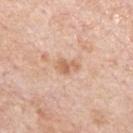No biopsy was performed on this lesion — it was imaged during a full skin examination and was not determined to be concerning. The subject is a male in their 70s. Imaged with white-light lighting. A region of skin cropped from a whole-body photographic capture, roughly 15 mm wide. Automated image analysis of the tile measured a within-lesion color-variation index near 2.5/10. The lesion is located on the front of the torso.